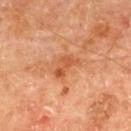| key | value |
|---|---|
| follow-up | imaged on a skin check; not biopsied |
| illumination | cross-polarized illumination |
| subject | male, aged 63 to 67 |
| image source | ~15 mm crop, total-body skin-cancer survey |
| location | the upper back |